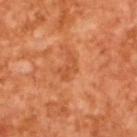Impression:
Recorded during total-body skin imaging; not selected for excision or biopsy.
Acquisition and patient details:
An algorithmic analysis of the crop reported an area of roughly 3.5 mm², an eccentricity of roughly 0.85, and a symmetry-axis asymmetry near 0.45. The analysis additionally found a mean CIELAB color near L≈52 a*≈30 b*≈41, roughly 7 lightness units darker than nearby skin, and a normalized border contrast of about 5. The analysis additionally found a classifier nevus-likeness of about 15/100 and a detector confidence of about 100 out of 100 that the crop contains a lesion. About 3 mm across. The tile uses cross-polarized illumination. The patient is a male aged around 65. A 15 mm close-up extracted from a 3D total-body photography capture.